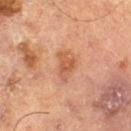Part of a total-body skin-imaging series; this lesion was reviewed on a skin check and was not flagged for biopsy. The lesion is on the left thigh. A region of skin cropped from a whole-body photographic capture, roughly 15 mm wide. This is a cross-polarized tile. The total-body-photography lesion software estimated a footprint of about 4.5 mm², an eccentricity of roughly 0.85, and a shape-asymmetry score of about 0.6 (0 = symmetric). The software also gave a lesion color around L≈45 a*≈22 b*≈30 in CIELAB, a lesion–skin lightness drop of about 8, and a normalized lesion–skin contrast near 6.5. The software also gave a border-irregularity index near 6/10, internal color variation of about 2 on a 0–10 scale, and radial color variation of about 0.5. The analysis additionally found a nevus-likeness score of about 20/100. The patient is a male aged around 70.